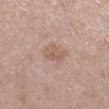Captured during whole-body skin photography for melanoma surveillance; the lesion was not biopsied. Located on the right lower leg. A 15 mm close-up extracted from a 3D total-body photography capture. Captured under white-light illumination. A female subject roughly 60 years of age.